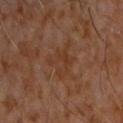Imaged during a routine full-body skin examination; the lesion was not biopsied and no histopathology is available. Cropped from a whole-body photographic skin survey; the tile spans about 15 mm. An algorithmic analysis of the crop reported a footprint of about 7.5 mm², a shape eccentricity near 0.5, and a symmetry-axis asymmetry near 0.5. The analysis additionally found a mean CIELAB color near L≈33 a*≈19 b*≈28, a lesion–skin lightness drop of about 4, and a lesion-to-skin contrast of about 5.5 (normalized; higher = more distinct). It also reported a within-lesion color-variation index near 2/10 and radial color variation of about 0.5. The analysis additionally found a nevus-likeness score of about 0/100 and a detector confidence of about 100 out of 100 that the crop contains a lesion. Captured under cross-polarized illumination. The patient is a male aged around 60. The lesion is on the chest. The recorded lesion diameter is about 4 mm.